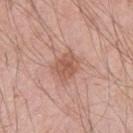Clinical impression: No biopsy was performed on this lesion — it was imaged during a full skin examination and was not determined to be concerning. Background: The recorded lesion diameter is about 3.5 mm. A lesion tile, about 15 mm wide, cut from a 3D total-body photograph. The tile uses white-light illumination. The patient is a male aged 43–47. The lesion is on the left upper arm.